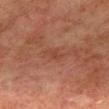follow-up = total-body-photography surveillance lesion; no biopsy
site = the mid back
acquisition = total-body-photography crop, ~15 mm field of view
patient = male, aged around 75